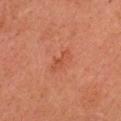Recorded during total-body skin imaging; not selected for excision or biopsy. A male patient about 45 years old. The tile uses cross-polarized illumination. The lesion-visualizer software estimated a lesion area of about 3 mm², an eccentricity of roughly 0.9, and two-axis asymmetry of about 0.35. And it measured a lesion-to-skin contrast of about 5 (normalized; higher = more distinct). The software also gave a border-irregularity rating of about 4/10, a color-variation rating of about 0/10, and peripheral color asymmetry of about 0. The analysis additionally found a nevus-likeness score of about 5/100 and a lesion-detection confidence of about 100/100. A close-up tile cropped from a whole-body skin photograph, about 15 mm across. The lesion is located on the head or neck.